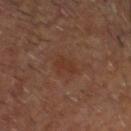Impression:
No biopsy was performed on this lesion — it was imaged during a full skin examination and was not determined to be concerning.
Background:
A 15 mm close-up tile from a total-body photography series done for melanoma screening. Automated image analysis of the tile measured a footprint of about 4 mm² and a shape-asymmetry score of about 0.3 (0 = symmetric). And it measured about 5 CIELAB-L* units darker than the surrounding skin and a normalized lesion–skin contrast near 5.5. It also reported a nevus-likeness score of about 0/100 and lesion-presence confidence of about 100/100. Located on the head or neck. A male patient, aged 58–62. Approximately 3 mm at its widest.